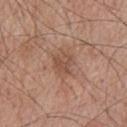The lesion was tiled from a total-body skin photograph and was not biopsied. Captured under white-light illumination. An algorithmic analysis of the crop reported a lesion color around L≈50 a*≈20 b*≈28 in CIELAB and a lesion–skin lightness drop of about 8. This image is a 15 mm lesion crop taken from a total-body photograph. Located on the upper back. The recorded lesion diameter is about 2.5 mm. A male subject aged approximately 65.